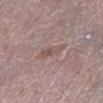biopsy status: no biopsy performed (imaged during a skin exam) | patient: male, about 65 years old | tile lighting: white-light | anatomic site: the left lower leg | automated metrics: a nevus-likeness score of about 0/100 | imaging modality: total-body-photography crop, ~15 mm field of view | diameter: ~3.5 mm (longest diameter).